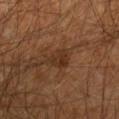notes = catalogued during a skin exam; not biopsied | subject = male, about 45 years old | TBP lesion metrics = a border-irregularity index near 4/10, a color-variation rating of about 3/10, and a peripheral color-asymmetry measure near 1; an automated nevus-likeness rating near 0 out of 100 and a lesion-detection confidence of about 100/100 | lighting = cross-polarized | image source = ~15 mm tile from a whole-body skin photo | site = the left forearm | diameter = ~3 mm (longest diameter).